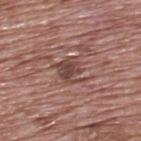No biopsy was performed on this lesion — it was imaged during a full skin examination and was not determined to be concerning. A male patient in their 70s. A lesion tile, about 15 mm wide, cut from a 3D total-body photograph. About 3.5 mm across. On the upper back.